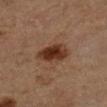The lesion was photographed on a routine skin check and not biopsied; there is no pathology result. A male patient aged approximately 85 or older. Cropped from a total-body skin-imaging series; the visible field is about 15 mm. Captured under cross-polarized illumination. The recorded lesion diameter is about 4 mm. The lesion is on the right lower leg.